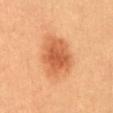Recorded during total-body skin imaging; not selected for excision or biopsy. On the front of the torso. The lesion's longest dimension is about 5 mm. A 15 mm close-up tile from a total-body photography series done for melanoma screening. A female patient, roughly 30 years of age. The tile uses cross-polarized illumination. The lesion-visualizer software estimated a border-irregularity rating of about 2.5/10, internal color variation of about 3.5 on a 0–10 scale, and peripheral color asymmetry of about 1. And it measured a detector confidence of about 100 out of 100 that the crop contains a lesion.